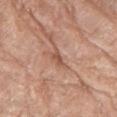No biopsy was performed on this lesion — it was imaged during a full skin examination and was not determined to be concerning. About 3 mm across. This image is a 15 mm lesion crop taken from a total-body photograph. This is a white-light tile. A female patient aged approximately 75. From the arm. An algorithmic analysis of the crop reported a border-irregularity index near 4.5/10, internal color variation of about 0 on a 0–10 scale, and peripheral color asymmetry of about 0. It also reported an automated nevus-likeness rating near 0 out of 100 and lesion-presence confidence of about 65/100.